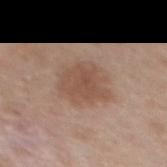Assessment:
This lesion was catalogued during total-body skin photography and was not selected for biopsy.